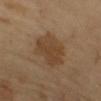Case summary:
* image · ~15 mm crop, total-body skin-cancer survey
* illumination · cross-polarized illumination
* patient · male, approximately 65 years of age
* automated lesion analysis · peripheral color asymmetry of about 0.5; a classifier nevus-likeness of about 35/100